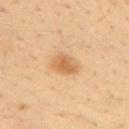Clinical impression:
Captured during whole-body skin photography for melanoma surveillance; the lesion was not biopsied.
Context:
The lesion is located on the back. The patient is a male in their mid-30s. A lesion tile, about 15 mm wide, cut from a 3D total-body photograph.